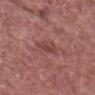Captured during whole-body skin photography for melanoma surveillance; the lesion was not biopsied.
An algorithmic analysis of the crop reported a footprint of about 4.5 mm², an outline eccentricity of about 0.85 (0 = round, 1 = elongated), and a shape-asymmetry score of about 0.3 (0 = symmetric). The analysis additionally found border irregularity of about 3.5 on a 0–10 scale, a within-lesion color-variation index near 2/10, and a peripheral color-asymmetry measure near 0.5.
A male patient, aged 68 to 72.
Cropped from a total-body skin-imaging series; the visible field is about 15 mm.
The lesion is on the head or neck.
The lesion's longest dimension is about 3.5 mm.